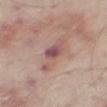<tbp_lesion>
  <biopsy_status>not biopsied; imaged during a skin examination</biopsy_status>
  <site>right thigh</site>
  <image>
    <source>total-body photography crop</source>
    <field_of_view_mm>15</field_of_view_mm>
  </image>
  <automated_metrics>
    <cielab_L>54</cielab_L>
    <cielab_a>21</cielab_a>
    <cielab_b>21</cielab_b>
    <vs_skin_darker_L>10.0</vs_skin_darker_L>
    <vs_skin_contrast_norm>7.5</vs_skin_contrast_norm>
    <nevus_likeness_0_100>0</nevus_likeness_0_100>
    <lesion_detection_confidence_0_100>100</lesion_detection_confidence_0_100>
  </automated_metrics>
  <patient>
    <sex>male</sex>
    <age_approx>65</age_approx>
  </patient>
  <lighting>white-light</lighting>
</tbp_lesion>